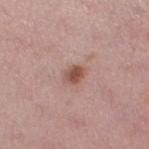Assessment: The lesion was tiled from a total-body skin photograph and was not biopsied. Image and clinical context: A female patient, about 50 years old. From the left thigh. About 2.5 mm across. A 15 mm close-up extracted from a 3D total-body photography capture.